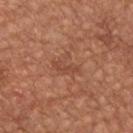workup: catalogued during a skin exam; not biopsied
acquisition: total-body-photography crop, ~15 mm field of view
body site: the front of the torso
patient: male, aged 63 to 67